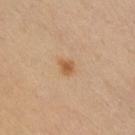Recorded during total-body skin imaging; not selected for excision or biopsy. A male patient, approximately 30 years of age. A close-up tile cropped from a whole-body skin photograph, about 15 mm across. This is a cross-polarized tile. The lesion is located on the front of the torso.